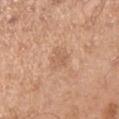Background:
Measured at roughly 3 mm in maximum diameter. Automated image analysis of the tile measured roughly 7 lightness units darker than nearby skin. The analysis additionally found a border-irregularity index near 2.5/10, a within-lesion color-variation index near 2/10, and a peripheral color-asymmetry measure near 0.5. The software also gave a classifier nevus-likeness of about 0/100 and a detector confidence of about 100 out of 100 that the crop contains a lesion. Located on the right upper arm. Cropped from a total-body skin-imaging series; the visible field is about 15 mm. A male patient, roughly 55 years of age.